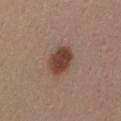{"biopsy_status": "not biopsied; imaged during a skin examination", "lighting": "white-light", "patient": {"sex": "female", "age_approx": 30}, "site": "mid back", "image": {"source": "total-body photography crop", "field_of_view_mm": 15}, "automated_metrics": {"eccentricity": 0.7, "shape_asymmetry": 0.15}}